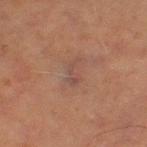Q: Was this lesion biopsied?
A: catalogued during a skin exam; not biopsied
Q: Where on the body is the lesion?
A: the right thigh
Q: Patient demographics?
A: male, aged approximately 70
Q: What is the imaging modality?
A: 15 mm crop, total-body photography
Q: What is the lesion's diameter?
A: ~3 mm (longest diameter)
Q: What did automated image analysis measure?
A: an area of roughly 3 mm² and a shape eccentricity near 0.85; an automated nevus-likeness rating near 0 out of 100 and lesion-presence confidence of about 100/100
Q: What lighting was used for the tile?
A: cross-polarized